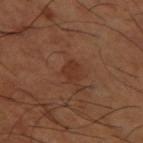No biopsy was performed on this lesion — it was imaged during a full skin examination and was not determined to be concerning.
Cropped from a whole-body photographic skin survey; the tile spans about 15 mm.
A male patient, aged approximately 65.
Longest diameter approximately 2.5 mm.
Captured under cross-polarized illumination.
The lesion is on the left thigh.